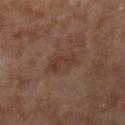workup: no biopsy performed (imaged during a skin exam)
lighting: cross-polarized illumination
image: 15 mm crop, total-body photography
TBP lesion metrics: an area of roughly 4.5 mm² and a symmetry-axis asymmetry near 0.4; a border-irregularity index near 5/10 and a peripheral color-asymmetry measure near 0.5; an automated nevus-likeness rating near 0 out of 100 and a lesion-detection confidence of about 100/100
size: ≈3.5 mm
site: the right lower leg
subject: female, roughly 60 years of age